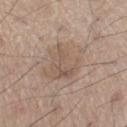This lesion was catalogued during total-body skin photography and was not selected for biopsy.
A male patient aged around 60.
Automated tile analysis of the lesion measured a lesion area of about 18 mm² and two-axis asymmetry of about 0.3. And it measured a mean CIELAB color near L≈56 a*≈15 b*≈26 and about 7 CIELAB-L* units darker than the surrounding skin. And it measured a nevus-likeness score of about 10/100 and lesion-presence confidence of about 100/100.
About 5.5 mm across.
A 15 mm close-up tile from a total-body photography series done for melanoma screening.
Captured under white-light illumination.
From the leg.